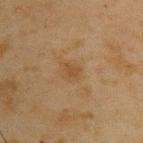Findings:
* notes: imaged on a skin check; not biopsied
* patient: male, aged approximately 45
* anatomic site: the upper back
* automated lesion analysis: a lesion area of about 4 mm², an outline eccentricity of about 0.6 (0 = round, 1 = elongated), and a symmetry-axis asymmetry near 0.2; a within-lesion color-variation index near 1/10 and radial color variation of about 0.5; an automated nevus-likeness rating near 0 out of 100 and a detector confidence of about 100 out of 100 that the crop contains a lesion
* acquisition: 15 mm crop, total-body photography
* illumination: cross-polarized